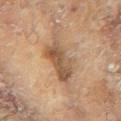Q: Who is the patient?
A: female, about 80 years old
Q: What is the anatomic site?
A: the right arm
Q: What is the imaging modality?
A: ~15 mm tile from a whole-body skin photo